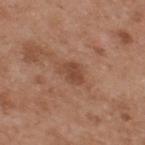Case summary:
- image source · ~15 mm crop, total-body skin-cancer survey
- subject · male, in their mid- to late 50s
- site · the back
- lesion diameter · ≈3 mm
- tile lighting · white-light
- TBP lesion metrics · an area of roughly 5 mm², an eccentricity of roughly 0.7, and a symmetry-axis asymmetry near 0.25